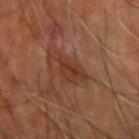follow-up=total-body-photography surveillance lesion; no biopsy | acquisition=~15 mm tile from a whole-body skin photo | patient=in their mid- to late 60s | body site=the right upper arm | size=~5 mm (longest diameter).